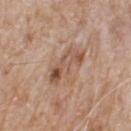Recorded during total-body skin imaging; not selected for excision or biopsy. Located on the upper back. The subject is a male in their 80s. A roughly 15 mm field-of-view crop from a total-body skin photograph. The tile uses white-light illumination.